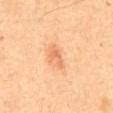Part of a total-body skin-imaging series; this lesion was reviewed on a skin check and was not flagged for biopsy.
This image is a 15 mm lesion crop taken from a total-body photograph.
A male subject, aged approximately 60.
From the abdomen.